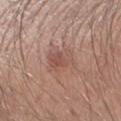Q: Was a biopsy performed?
A: no biopsy performed (imaged during a skin exam)
Q: Patient demographics?
A: male, approximately 30 years of age
Q: What did automated image analysis measure?
A: a lesion color around L≈52 a*≈21 b*≈25 in CIELAB, a lesion–skin lightness drop of about 8, and a lesion-to-skin contrast of about 5.5 (normalized; higher = more distinct); an automated nevus-likeness rating near 20 out of 100
Q: How was this image acquired?
A: total-body-photography crop, ~15 mm field of view
Q: How was the tile lit?
A: white-light illumination
Q: Where on the body is the lesion?
A: the right forearm
Q: What is the lesion's diameter?
A: ~3.5 mm (longest diameter)